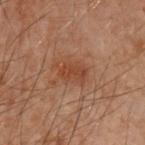Imaged during a routine full-body skin examination; the lesion was not biopsied and no histopathology is available.
On the back.
Automated image analysis of the tile measured a lesion–skin lightness drop of about 6 and a normalized border contrast of about 6. The software also gave a border-irregularity index near 2/10, a within-lesion color-variation index near 2/10, and peripheral color asymmetry of about 1.
Longest diameter approximately 3 mm.
This image is a 15 mm lesion crop taken from a total-body photograph.
The tile uses cross-polarized illumination.
A male subject, roughly 50 years of age.